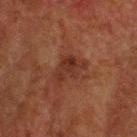| key | value |
|---|---|
| biopsy status | catalogued during a skin exam; not biopsied |
| subject | male, aged 58 to 62 |
| acquisition | ~15 mm crop, total-body skin-cancer survey |
| anatomic site | the head or neck |